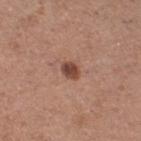Automated tile analysis of the lesion measured a lesion color around L≈46 a*≈22 b*≈27 in CIELAB and a lesion-to-skin contrast of about 9.5 (normalized; higher = more distinct). The software also gave a classifier nevus-likeness of about 95/100.
A 15 mm close-up tile from a total-body photography series done for melanoma screening.
The lesion is on the right thigh.
The patient is a female aged 28–32.
This is a white-light tile.
The lesion's longest dimension is about 2.5 mm.